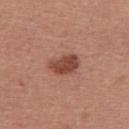The lesion was photographed on a routine skin check and not biopsied; there is no pathology result. The subject is a male aged 38–42. The lesion is on the upper back. The tile uses white-light illumination. The lesion-visualizer software estimated a footprint of about 7.5 mm², an eccentricity of roughly 0.8, and a shape-asymmetry score of about 0.25 (0 = symmetric). The software also gave a border-irregularity index near 2.5/10, a color-variation rating of about 2.5/10, and a peripheral color-asymmetry measure near 1. And it measured a lesion-detection confidence of about 100/100. Cropped from a whole-body photographic skin survey; the tile spans about 15 mm. The lesion's longest dimension is about 4 mm.